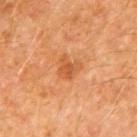patient — male, in their 60s | anatomic site — the right upper arm | image-analysis metrics — a footprint of about 5 mm², a shape eccentricity near 0.25, and a shape-asymmetry score of about 0.4 (0 = symmetric); border irregularity of about 4 on a 0–10 scale and peripheral color asymmetry of about 0.5; an automated nevus-likeness rating near 60 out of 100 | illumination — cross-polarized | diameter — ~2.5 mm (longest diameter) | imaging modality — total-body-photography crop, ~15 mm field of view.